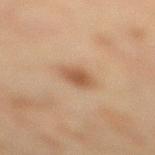Imaged during a routine full-body skin examination; the lesion was not biopsied and no histopathology is available.
The patient is a female aged around 55.
Longest diameter approximately 2.5 mm.
Captured under cross-polarized illumination.
A 15 mm close-up tile from a total-body photography series done for melanoma screening.
The lesion is on the right lower leg.